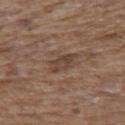follow-up: imaged on a skin check; not biopsied | TBP lesion metrics: an automated nevus-likeness rating near 0 out of 100 | subject: female, in their mid- to late 60s | lighting: white-light | diameter: ~3 mm (longest diameter) | image: ~15 mm crop, total-body skin-cancer survey | site: the upper back.